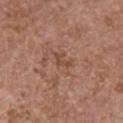- workup: total-body-photography surveillance lesion; no biopsy
- illumination: white-light
- image-analysis metrics: radial color variation of about 0
- acquisition: 15 mm crop, total-body photography
- lesion size: ≈2.5 mm
- subject: female, aged around 65
- location: the chest